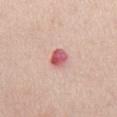No biopsy was performed on this lesion — it was imaged during a full skin examination and was not determined to be concerning.
On the chest.
Captured under white-light illumination.
About 2.5 mm across.
The subject is a male roughly 40 years of age.
A close-up tile cropped from a whole-body skin photograph, about 15 mm across.